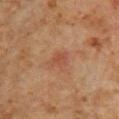biopsy status = total-body-photography surveillance lesion; no biopsy.